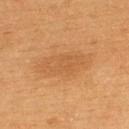Clinical impression: This lesion was catalogued during total-body skin photography and was not selected for biopsy. Background: The patient is a male about 60 years old. From the upper back. The lesion's longest dimension is about 6.5 mm. A region of skin cropped from a whole-body photographic capture, roughly 15 mm wide.